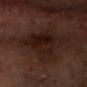workup=catalogued during a skin exam; not biopsied | diameter=≈7.5 mm | patient=male, aged 68–72 | tile lighting=cross-polarized illumination | site=the head or neck | acquisition=total-body-photography crop, ~15 mm field of view.